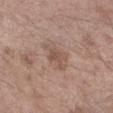patient:
  sex: male
  age_approx: 60
lighting: white-light
lesion_size:
  long_diameter_mm_approx: 3.5
automated_metrics:
  cielab_L: 52
  cielab_a: 17
  cielab_b: 25
  vs_skin_darker_L: 8.0
  vs_skin_contrast_norm: 5.5
  nevus_likeness_0_100: 0
  lesion_detection_confidence_0_100: 100
site: arm
image:
  source: total-body photography crop
  field_of_view_mm: 15